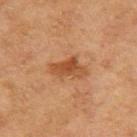Impression: Imaged during a routine full-body skin examination; the lesion was not biopsied and no histopathology is available. Acquisition and patient details: On the arm. An algorithmic analysis of the crop reported a mean CIELAB color near L≈44 a*≈22 b*≈35, roughly 10 lightness units darker than nearby skin, and a lesion-to-skin contrast of about 8 (normalized; higher = more distinct). The analysis additionally found a color-variation rating of about 3.5/10. It also reported an automated nevus-likeness rating near 20 out of 100 and lesion-presence confidence of about 100/100. The subject is a female about 60 years old. Measured at roughly 4 mm in maximum diameter. Cropped from a total-body skin-imaging series; the visible field is about 15 mm. The tile uses cross-polarized illumination.